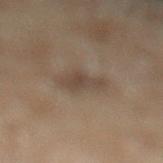<case>
<biopsy_status>not biopsied; imaged during a skin examination</biopsy_status>
<patient>
  <sex>male</sex>
  <age_approx>70</age_approx>
</patient>
<image>
  <source>total-body photography crop</source>
  <field_of_view_mm>15</field_of_view_mm>
</image>
<lesion_size>
  <long_diameter_mm_approx>3.0</long_diameter_mm_approx>
</lesion_size>
<site>leg</site>
</case>